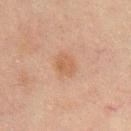Assessment:
The lesion was photographed on a routine skin check and not biopsied; there is no pathology result.
Context:
A lesion tile, about 15 mm wide, cut from a 3D total-body photograph. A male patient, aged 48–52. The lesion is located on the chest. Captured under cross-polarized illumination. Automated tile analysis of the lesion measured a border-irregularity index near 1.5/10, internal color variation of about 1.5 on a 0–10 scale, and a peripheral color-asymmetry measure near 0.5.